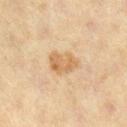Imaged during a routine full-body skin examination; the lesion was not biopsied and no histopathology is available. Located on the right lower leg. A 15 mm close-up extracted from a 3D total-body photography capture. The patient is a female aged around 40.